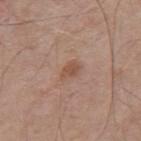Q: What lighting was used for the tile?
A: white-light
Q: What is the imaging modality?
A: total-body-photography crop, ~15 mm field of view
Q: Lesion location?
A: the upper back
Q: Who is the patient?
A: male, aged approximately 70